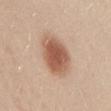biopsy status: no biopsy performed (imaged during a skin exam)
acquisition: 15 mm crop, total-body photography
body site: the lower back
diameter: about 5.5 mm
subject: female, about 20 years old